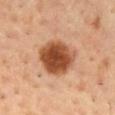automated metrics: a lesion color around L≈48 a*≈25 b*≈35 in CIELAB; lesion-presence confidence of about 100/100 | site: the back | lesion size: ≈5.5 mm | illumination: cross-polarized illumination | image source: total-body-photography crop, ~15 mm field of view | patient: male, aged 53 to 57.